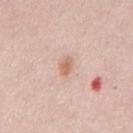{"site": "front of the torso", "patient": {"sex": "male", "age_approx": 50}, "lesion_size": {"long_diameter_mm_approx": 2.5}, "lighting": "white-light", "image": {"source": "total-body photography crop", "field_of_view_mm": 15}, "automated_metrics": {"area_mm2_approx": 3.5, "shape_asymmetry": 0.2, "cielab_L": 66, "cielab_a": 20, "cielab_b": 30, "vs_skin_darker_L": 9.0, "border_irregularity_0_10": 2.0, "color_variation_0_10": 3.0, "peripheral_color_asymmetry": 1.0, "nevus_likeness_0_100": 75, "lesion_detection_confidence_0_100": 100}}